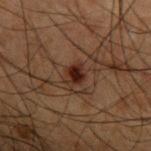Case summary:
* biopsy status — no biopsy performed (imaged during a skin exam)
* lesion size — ≈3 mm
* subject — male, about 50 years old
* automated metrics — a lesion area of about 4.5 mm², a shape eccentricity near 0.7, and a symmetry-axis asymmetry near 0.25; a lesion color around L≈20 a*≈16 b*≈20 in CIELAB and roughly 8 lightness units darker than nearby skin; an automated nevus-likeness rating near 90 out of 100 and lesion-presence confidence of about 100/100
* anatomic site — the left upper arm
* lighting — cross-polarized
* acquisition — 15 mm crop, total-body photography